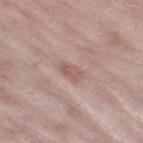Recorded during total-body skin imaging; not selected for excision or biopsy.
The patient is a female roughly 70 years of age.
Cropped from a whole-body photographic skin survey; the tile spans about 15 mm.
The lesion's longest dimension is about 3 mm.
An algorithmic analysis of the crop reported an area of roughly 3.5 mm², an eccentricity of roughly 0.85, and two-axis asymmetry of about 0.3. And it measured a lesion color around L≈56 a*≈19 b*≈23 in CIELAB, a lesion–skin lightness drop of about 8, and a normalized lesion–skin contrast near 5.5. The software also gave a nevus-likeness score of about 0/100 and lesion-presence confidence of about 100/100.
Located on the leg.
Captured under white-light illumination.